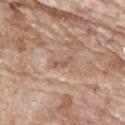<case>
  <biopsy_status>not biopsied; imaged during a skin examination</biopsy_status>
  <lighting>white-light</lighting>
  <image>
    <source>total-body photography crop</source>
    <field_of_view_mm>15</field_of_view_mm>
  </image>
  <patient>
    <sex>male</sex>
    <age_approx>65</age_approx>
  </patient>
  <lesion_size>
    <long_diameter_mm_approx>2.5</long_diameter_mm_approx>
  </lesion_size>
  <site>upper back</site>
</case>